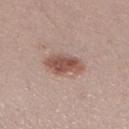Imaged during a routine full-body skin examination; the lesion was not biopsied and no histopathology is available. The lesion-visualizer software estimated a lesion area of about 8.5 mm² and an outline eccentricity of about 0.8 (0 = round, 1 = elongated). And it measured a border-irregularity index near 3/10, internal color variation of about 4 on a 0–10 scale, and a peripheral color-asymmetry measure near 1. On the left lower leg. A roughly 15 mm field-of-view crop from a total-body skin photograph. The tile uses white-light illumination. A female patient aged approximately 25.